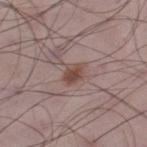No biopsy was performed on this lesion — it was imaged during a full skin examination and was not determined to be concerning. A male subject in their 50s. The lesion is on the right lower leg. This is a white-light tile. Approximately 2.5 mm at its widest. Cropped from a total-body skin-imaging series; the visible field is about 15 mm.